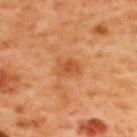Clinical impression: The lesion was tiled from a total-body skin photograph and was not biopsied. Image and clinical context: Longest diameter approximately 3 mm. A male patient aged around 50. Cropped from a total-body skin-imaging series; the visible field is about 15 mm. The lesion is located on the back.